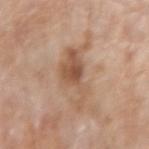Q: Is there a histopathology result?
A: total-body-photography surveillance lesion; no biopsy
Q: How was the tile lit?
A: white-light
Q: What kind of image is this?
A: ~15 mm tile from a whole-body skin photo
Q: What did automated image analysis measure?
A: a footprint of about 13 mm², an outline eccentricity of about 0.85 (0 = round, 1 = elongated), and a symmetry-axis asymmetry near 0.5; an average lesion color of about L≈55 a*≈19 b*≈31 (CIELAB) and a normalized lesion–skin contrast near 7; border irregularity of about 8 on a 0–10 scale, a color-variation rating of about 3.5/10, and peripheral color asymmetry of about 1
Q: How large is the lesion?
A: ≈6 mm
Q: What is the anatomic site?
A: the left forearm
Q: Who is the patient?
A: female, about 75 years old